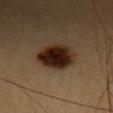Q: Was a biopsy performed?
A: total-body-photography surveillance lesion; no biopsy
Q: Lesion location?
A: the head or neck
Q: How was this image acquired?
A: 15 mm crop, total-body photography
Q: What is the lesion's diameter?
A: ~5 mm (longest diameter)
Q: What did automated image analysis measure?
A: a mean CIELAB color near L≈20 a*≈15 b*≈22 and a normalized lesion–skin contrast near 16.5; border irregularity of about 1.5 on a 0–10 scale, internal color variation of about 6.5 on a 0–10 scale, and a peripheral color-asymmetry measure near 1.5; a classifier nevus-likeness of about 100/100 and a lesion-detection confidence of about 100/100
Q: Who is the patient?
A: female, aged approximately 30